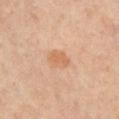Imaged during a routine full-body skin examination; the lesion was not biopsied and no histopathology is available. An algorithmic analysis of the crop reported border irregularity of about 3 on a 0–10 scale and peripheral color asymmetry of about 0.5. And it measured a nevus-likeness score of about 15/100. The subject is a male aged around 65. From the chest. This image is a 15 mm lesion crop taken from a total-body photograph. About 2.5 mm across. Imaged with cross-polarized lighting.